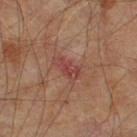No biopsy was performed on this lesion — it was imaged during a full skin examination and was not determined to be concerning. On the leg. A male subject, aged 58 to 62. A lesion tile, about 15 mm wide, cut from a 3D total-body photograph. An algorithmic analysis of the crop reported a mean CIELAB color near L≈41 a*≈25 b*≈24, about 7 CIELAB-L* units darker than the surrounding skin, and a normalized lesion–skin contrast near 6. And it measured a border-irregularity index near 3.5/10 and peripheral color asymmetry of about 1. This is a cross-polarized tile.